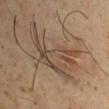Case summary:
* notes: total-body-photography surveillance lesion; no biopsy
* anatomic site: the left upper arm
* illumination: cross-polarized illumination
* lesion diameter: about 8 mm
* patient: male, aged 48 to 52
* automated metrics: a lesion–skin lightness drop of about 8; border irregularity of about 10 on a 0–10 scale, internal color variation of about 4.5 on a 0–10 scale, and peripheral color asymmetry of about 1.5; an automated nevus-likeness rating near 25 out of 100
* imaging modality: total-body-photography crop, ~15 mm field of view